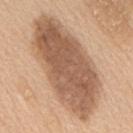This lesion was catalogued during total-body skin photography and was not selected for biopsy. A male subject in their 60s. Cropped from a total-body skin-imaging series; the visible field is about 15 mm. The lesion is on the back.